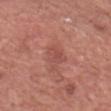Clinical impression: No biopsy was performed on this lesion — it was imaged during a full skin examination and was not determined to be concerning. Clinical summary: Captured under white-light illumination. About 2.5 mm across. Automated tile analysis of the lesion measured a border-irregularity rating of about 2.5/10 and a color-variation rating of about 1/10. The software also gave lesion-presence confidence of about 100/100. A male subject in their 80s. A region of skin cropped from a whole-body photographic capture, roughly 15 mm wide. The lesion is located on the head or neck.